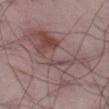Q: Was a biopsy performed?
A: catalogued during a skin exam; not biopsied